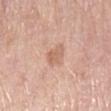Q: Is there a histopathology result?
A: imaged on a skin check; not biopsied
Q: What is the anatomic site?
A: the right lower leg
Q: What kind of image is this?
A: 15 mm crop, total-body photography
Q: Patient demographics?
A: female, aged approximately 65